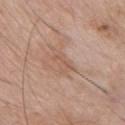  biopsy_status: not biopsied; imaged during a skin examination